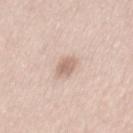Part of a total-body skin-imaging series; this lesion was reviewed on a skin check and was not flagged for biopsy. The total-body-photography lesion software estimated a footprint of about 4 mm² and an outline eccentricity of about 0.75 (0 = round, 1 = elongated). The software also gave a lesion color around L≈65 a*≈17 b*≈26 in CIELAB, a lesion–skin lightness drop of about 11, and a normalized border contrast of about 6.5. Approximately 2.5 mm at its widest. A region of skin cropped from a whole-body photographic capture, roughly 15 mm wide. The tile uses white-light illumination. A male patient in their mid-40s. The lesion is located on the mid back.